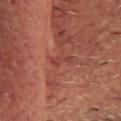{"biopsy_status": "not biopsied; imaged during a skin examination", "image": {"source": "total-body photography crop", "field_of_view_mm": 15}, "site": "head or neck", "lighting": "cross-polarized", "patient": {"sex": "male", "age_approx": 60}, "lesion_size": {"long_diameter_mm_approx": 3.0}}